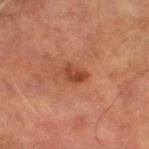This lesion was catalogued during total-body skin photography and was not selected for biopsy.
The lesion is located on the right thigh.
This is a cross-polarized tile.
The lesion's longest dimension is about 3 mm.
A 15 mm crop from a total-body photograph taken for skin-cancer surveillance.
A male subject aged approximately 75.